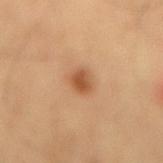<lesion>
  <biopsy_status>not biopsied; imaged during a skin examination</biopsy_status>
  <patient>
    <sex>male</sex>
    <age_approx>60</age_approx>
  </patient>
  <site>lower back</site>
  <lesion_size>
    <long_diameter_mm_approx>2.5</long_diameter_mm_approx>
  </lesion_size>
  <lighting>cross-polarized</lighting>
  <image>
    <source>total-body photography crop</source>
    <field_of_view_mm>15</field_of_view_mm>
  </image>
  <automated_metrics>
    <eccentricity>0.65</eccentricity>
    <shape_asymmetry>0.25</shape_asymmetry>
    <vs_skin_darker_L>11.0</vs_skin_darker_L>
  </automated_metrics>
</lesion>